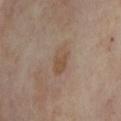No biopsy was performed on this lesion — it was imaged during a full skin examination and was not determined to be concerning. A female subject in their mid-50s. The lesion is on the left lower leg. A lesion tile, about 15 mm wide, cut from a 3D total-body photograph.